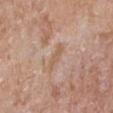This lesion was catalogued during total-body skin photography and was not selected for biopsy. Approximately 3 mm at its widest. From the left lower leg. Automated tile analysis of the lesion measured an outline eccentricity of about 0.95 (0 = round, 1 = elongated) and a shape-asymmetry score of about 0.45 (0 = symmetric). It also reported a border-irregularity index near 5/10. A male patient aged around 75. The tile uses white-light illumination. A lesion tile, about 15 mm wide, cut from a 3D total-body photograph.